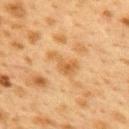Part of a total-body skin-imaging series; this lesion was reviewed on a skin check and was not flagged for biopsy.
On the upper back.
The recorded lesion diameter is about 4 mm.
The patient is a female approximately 40 years of age.
The lesion-visualizer software estimated a mean CIELAB color near L≈51 a*≈18 b*≈38 and about 7 CIELAB-L* units darker than the surrounding skin. It also reported a border-irregularity rating of about 5/10 and internal color variation of about 3.5 on a 0–10 scale. The software also gave a classifier nevus-likeness of about 0/100 and a detector confidence of about 100 out of 100 that the crop contains a lesion.
A 15 mm close-up extracted from a 3D total-body photography capture.